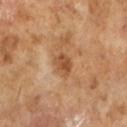notes: total-body-photography surveillance lesion; no biopsy
subject: male, aged around 65
imaging modality: 15 mm crop, total-body photography
lighting: cross-polarized
lesion diameter: about 3 mm